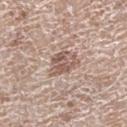– follow-up · total-body-photography surveillance lesion; no biopsy
– patient · female, in their mid-70s
– image-analysis metrics · a lesion area of about 8.5 mm² and a symmetry-axis asymmetry near 0.25; an automated nevus-likeness rating near 0 out of 100 and lesion-presence confidence of about 85/100
– imaging modality · ~15 mm crop, total-body skin-cancer survey
– diameter · ≈4 mm
– anatomic site · the right lower leg